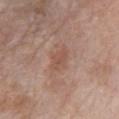Captured during whole-body skin photography for melanoma surveillance; the lesion was not biopsied.